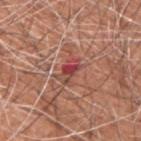Findings:
* biopsy status · no biopsy performed (imaged during a skin exam)
* imaging modality · 15 mm crop, total-body photography
* automated lesion analysis · a footprint of about 4.5 mm², a shape eccentricity near 0.85, and two-axis asymmetry of about 0.25
* illumination · white-light
* body site · the upper back
* subject · male, in their 60s